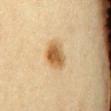The lesion was tiled from a total-body skin photograph and was not biopsied. The lesion is on the front of the torso. The lesion-visualizer software estimated a lesion area of about 8.5 mm², an eccentricity of roughly 0.7, and a shape-asymmetry score of about 0.2 (0 = symmetric). Approximately 4 mm at its widest. A 15 mm close-up tile from a total-body photography series done for melanoma screening. A female subject in their mid- to late 50s. This is a cross-polarized tile.